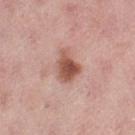Q: Is there a histopathology result?
A: catalogued during a skin exam; not biopsied
Q: Lesion location?
A: the left thigh
Q: How was the tile lit?
A: white-light
Q: How was this image acquired?
A: total-body-photography crop, ~15 mm field of view
Q: What did automated image analysis measure?
A: a mean CIELAB color near L≈53 a*≈24 b*≈28, a lesion–skin lightness drop of about 14, and a normalized border contrast of about 9.5; a within-lesion color-variation index near 5.5/10; an automated nevus-likeness rating near 90 out of 100
Q: What is the lesion's diameter?
A: ~3.5 mm (longest diameter)
Q: Who is the patient?
A: female, approximately 50 years of age